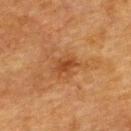This lesion was catalogued during total-body skin photography and was not selected for biopsy.
On the upper back.
A roughly 15 mm field-of-view crop from a total-body skin photograph.
A female patient about 55 years old.